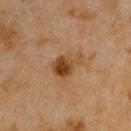biopsy status — total-body-photography surveillance lesion; no biopsy
anatomic site — the upper back
TBP lesion metrics — a lesion color around L≈36 a*≈17 b*≈30 in CIELAB, a lesion–skin lightness drop of about 9, and a lesion-to-skin contrast of about 8.5 (normalized; higher = more distinct); a color-variation rating of about 6/10 and a peripheral color-asymmetry measure near 2; a nevus-likeness score of about 85/100 and a detector confidence of about 100 out of 100 that the crop contains a lesion
illumination — cross-polarized illumination
size — about 4 mm
patient — male, in their mid-40s
image — 15 mm crop, total-body photography